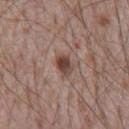The lesion was tiled from a total-body skin photograph and was not biopsied.
The patient is a male aged 68–72.
A 15 mm crop from a total-body photograph taken for skin-cancer surveillance.
The lesion is located on the abdomen.